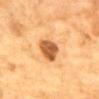Q: Was this lesion biopsied?
A: total-body-photography surveillance lesion; no biopsy
Q: Patient demographics?
A: male, aged around 85 or older
Q: Lesion location?
A: the chest
Q: What is the imaging modality?
A: ~15 mm crop, total-body skin-cancer survey